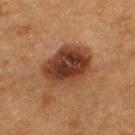  biopsy_status: not biopsied; imaged during a skin examination
  site: upper back
  lighting: cross-polarized
  patient:
    sex: male
    age_approx: 50
  image:
    source: total-body photography crop
    field_of_view_mm: 15
  lesion_size:
    long_diameter_mm_approx: 6.0